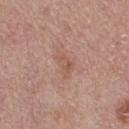Assessment:
Part of a total-body skin-imaging series; this lesion was reviewed on a skin check and was not flagged for biopsy.
Clinical summary:
A female patient, in their mid- to late 60s. An algorithmic analysis of the crop reported an area of roughly 4.5 mm², an eccentricity of roughly 0.8, and a symmetry-axis asymmetry near 0.45. Located on the right thigh. A 15 mm close-up extracted from a 3D total-body photography capture. Captured under white-light illumination. Approximately 3 mm at its widest.